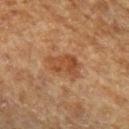| feature | finding |
|---|---|
| workup | imaged on a skin check; not biopsied |
| location | the left forearm |
| patient | male, aged around 65 |
| acquisition | total-body-photography crop, ~15 mm field of view |